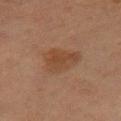{
  "lighting": "cross-polarized",
  "site": "right thigh",
  "image": {
    "source": "total-body photography crop",
    "field_of_view_mm": 15
  },
  "patient": {
    "sex": "female",
    "age_approx": 40
  },
  "lesion_size": {
    "long_diameter_mm_approx": 4.5
  }
}